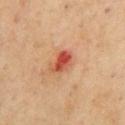Notes:
- notes — no biopsy performed (imaged during a skin exam)
- acquisition — ~15 mm crop, total-body skin-cancer survey
- patient — male, aged 68 to 72
- body site — the chest